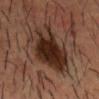<record>
  <biopsy_status>not biopsied; imaged during a skin examination</biopsy_status>
  <patient>
    <sex>male</sex>
    <age_approx>35</age_approx>
  </patient>
  <lesion_size>
    <long_diameter_mm_approx>9.5</long_diameter_mm_approx>
  </lesion_size>
  <automated_metrics>
    <area_mm2_approx>27.0</area_mm2_approx>
    <eccentricity>0.9</eccentricity>
    <border_irregularity_0_10>5.0</border_irregularity_0_10>
    <color_variation_0_10>6.5</color_variation_0_10>
    <nevus_likeness_0_100>95</nevus_likeness_0_100>
    <lesion_detection_confidence_0_100>95</lesion_detection_confidence_0_100>
  </automated_metrics>
  <image>
    <source>total-body photography crop</source>
    <field_of_view_mm>15</field_of_view_mm>
  </image>
  <lighting>cross-polarized</lighting>
  <site>head or neck</site>
</record>